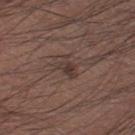Imaged during a routine full-body skin examination; the lesion was not biopsied and no histopathology is available. A region of skin cropped from a whole-body photographic capture, roughly 15 mm wide. The recorded lesion diameter is about 3 mm. A male subject, aged approximately 40. Located on the left thigh. The lesion-visualizer software estimated an average lesion color of about L≈36 a*≈15 b*≈19 (CIELAB) and a lesion-to-skin contrast of about 7 (normalized; higher = more distinct). And it measured a border-irregularity index near 4/10, internal color variation of about 3.5 on a 0–10 scale, and radial color variation of about 1. The analysis additionally found a classifier nevus-likeness of about 30/100 and a detector confidence of about 100 out of 100 that the crop contains a lesion.